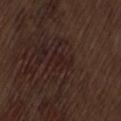Impression:
The lesion was tiled from a total-body skin photograph and was not biopsied.
Acquisition and patient details:
The subject is a male aged approximately 70. The lesion is on the lower back. Imaged with white-light lighting. This image is a 15 mm lesion crop taken from a total-body photograph. About 3 mm across.